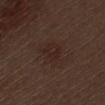| feature | finding |
|---|---|
| workup | catalogued during a skin exam; not biopsied |
| image source | total-body-photography crop, ~15 mm field of view |
| patient | male, about 70 years old |
| TBP lesion metrics | an eccentricity of roughly 0.6 and two-axis asymmetry of about 0.2; roughly 4 lightness units darker than nearby skin; internal color variation of about 2.5 on a 0–10 scale and peripheral color asymmetry of about 1; a lesion-detection confidence of about 100/100 |
| site | the left thigh |
| illumination | white-light |